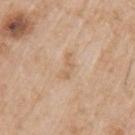The lesion was tiled from a total-body skin photograph and was not biopsied. A male subject approximately 65 years of age. A 15 mm crop from a total-body photograph taken for skin-cancer surveillance. Approximately 3 mm at its widest. On the arm.